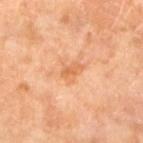<case>
  <biopsy_status>not biopsied; imaged during a skin examination</biopsy_status>
  <image>
    <source>total-body photography crop</source>
    <field_of_view_mm>15</field_of_view_mm>
  </image>
  <site>left lower leg</site>
  <automated_metrics>
    <area_mm2_approx>4.0</area_mm2_approx>
    <eccentricity>0.75</eccentricity>
    <cielab_L>63</cielab_L>
    <cielab_a>26</cielab_a>
    <cielab_b>40</cielab_b>
    <vs_skin_darker_L>8.0</vs_skin_darker_L>
    <vs_skin_contrast_norm>6.0</vs_skin_contrast_norm>
    <border_irregularity_0_10>3.5</border_irregularity_0_10>
    <nevus_likeness_0_100>0</nevus_likeness_0_100>
    <lesion_detection_confidence_0_100>100</lesion_detection_confidence_0_100>
  </automated_metrics>
  <patient>
    <sex>female</sex>
    <age_approx>70</age_approx>
  </patient>
  <lesion_size>
    <long_diameter_mm_approx>2.5</long_diameter_mm_approx>
  </lesion_size>
</case>